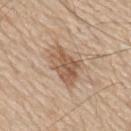<lesion>
<biopsy_status>not biopsied; imaged during a skin examination</biopsy_status>
<lighting>white-light</lighting>
<patient>
  <sex>male</sex>
  <age_approx>80</age_approx>
</patient>
<image>
  <source>total-body photography crop</source>
  <field_of_view_mm>15</field_of_view_mm>
</image>
<site>mid back</site>
<lesion_size>
  <long_diameter_mm_approx>5.0</long_diameter_mm_approx>
</lesion_size>
</lesion>